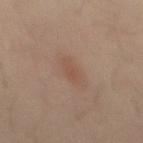Part of a total-body skin-imaging series; this lesion was reviewed on a skin check and was not flagged for biopsy.
The subject is a male aged 48 to 52.
The lesion is located on the mid back.
This is a cross-polarized tile.
This image is a 15 mm lesion crop taken from a total-body photograph.